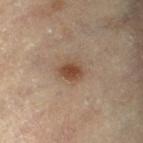Image and clinical context:
This is a cross-polarized tile. This image is a 15 mm lesion crop taken from a total-body photograph. The lesion-visualizer software estimated a border-irregularity index near 1.5/10 and peripheral color asymmetry of about 1. The software also gave a classifier nevus-likeness of about 95/100. Approximately 3 mm at its widest. A male patient aged 83 or older. On the left thigh.